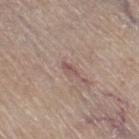{"biopsy_status": "not biopsied; imaged during a skin examination", "site": "right thigh", "automated_metrics": {"vs_skin_darker_L": 8.0, "vs_skin_contrast_norm": 6.0}, "image": {"source": "total-body photography crop", "field_of_view_mm": 15}, "patient": {"sex": "male", "age_approx": 65}}